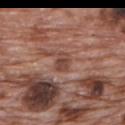<record>
  <biopsy_status>not biopsied; imaged during a skin examination</biopsy_status>
  <image>
    <source>total-body photography crop</source>
    <field_of_view_mm>15</field_of_view_mm>
  </image>
  <lesion_size>
    <long_diameter_mm_approx>3.0</long_diameter_mm_approx>
  </lesion_size>
  <automated_metrics>
    <area_mm2_approx>4.5</area_mm2_approx>
    <eccentricity>0.65</eccentricity>
    <shape_asymmetry>0.3</shape_asymmetry>
    <cielab_L>45</cielab_L>
    <cielab_a>21</cielab_a>
    <cielab_b>26</cielab_b>
    <vs_skin_darker_L>8.0</vs_skin_darker_L>
    <vs_skin_contrast_norm>6.0</vs_skin_contrast_norm>
    <border_irregularity_0_10>2.5</border_irregularity_0_10>
    <color_variation_0_10>3.0</color_variation_0_10>
    <peripheral_color_asymmetry>1.0</peripheral_color_asymmetry>
  </automated_metrics>
  <patient>
    <sex>male</sex>
    <age_approx>70</age_approx>
  </patient>
  <lighting>white-light</lighting>
  <site>back</site>
</record>